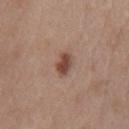lesion_size:
  long_diameter_mm_approx: 3.0
automated_metrics:
  area_mm2_approx: 4.5
  border_irregularity_0_10: 2.5
  color_variation_0_10: 3.0
  peripheral_color_asymmetry: 1.0
patient:
  sex: female
  age_approx: 40
site: front of the torso
image:
  source: total-body photography crop
  field_of_view_mm: 15
lighting: white-light